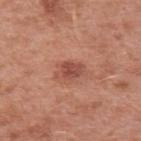Q: Is there a histopathology result?
A: total-body-photography surveillance lesion; no biopsy
Q: What are the patient's age and sex?
A: female, aged approximately 40
Q: What is the lesion's diameter?
A: about 3 mm
Q: How was the tile lit?
A: white-light illumination
Q: How was this image acquired?
A: 15 mm crop, total-body photography
Q: Lesion location?
A: the left upper arm